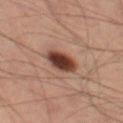| field | value |
|---|---|
| workup | catalogued during a skin exam; not biopsied |
| body site | the leg |
| tile lighting | cross-polarized illumination |
| patient | male, aged approximately 60 |
| acquisition | ~15 mm crop, total-body skin-cancer survey |